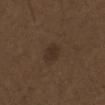Notes:
– follow-up · catalogued during a skin exam; not biopsied